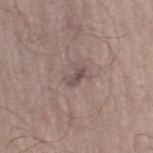{"biopsy_status": "not biopsied; imaged during a skin examination", "site": "right lower leg", "patient": {"sex": "male", "age_approx": 25}, "image": {"source": "total-body photography crop", "field_of_view_mm": 15}, "lighting": "white-light", "lesion_size": {"long_diameter_mm_approx": 2.5}, "automated_metrics": {"area_mm2_approx": 3.0, "eccentricity": 0.85, "shape_asymmetry": 0.3, "border_irregularity_0_10": 3.0, "color_variation_0_10": 0.5, "peripheral_color_asymmetry": 0.0}}